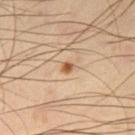Impression:
Captured during whole-body skin photography for melanoma surveillance; the lesion was not biopsied.
Context:
The lesion is located on the right thigh. The recorded lesion diameter is about 1.5 mm. This is a cross-polarized tile. The lesion-visualizer software estimated a shape eccentricity near 0.55 and two-axis asymmetry of about 0.2. It also reported an average lesion color of about L≈57 a*≈21 b*≈37 (CIELAB), a lesion–skin lightness drop of about 13, and a lesion-to-skin contrast of about 9 (normalized; higher = more distinct). It also reported a border-irregularity rating of about 1.5/10 and a peripheral color-asymmetry measure near 0.5. A 15 mm crop from a total-body photograph taken for skin-cancer surveillance. The patient is a male in their 40s.